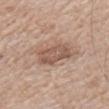Part of a total-body skin-imaging series; this lesion was reviewed on a skin check and was not flagged for biopsy. A region of skin cropped from a whole-body photographic capture, roughly 15 mm wide. Imaged with white-light lighting. The lesion is on the left upper arm. A male subject aged around 70.